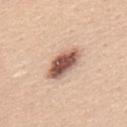No biopsy was performed on this lesion — it was imaged during a full skin examination and was not determined to be concerning.
From the mid back.
The tile uses white-light illumination.
Approximately 4.5 mm at its widest.
Cropped from a total-body skin-imaging series; the visible field is about 15 mm.
A male subject, in their mid- to late 40s.